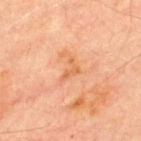Q: Was a biopsy performed?
A: imaged on a skin check; not biopsied
Q: What are the patient's age and sex?
A: male, roughly 70 years of age
Q: How was this image acquired?
A: ~15 mm tile from a whole-body skin photo
Q: Illumination type?
A: cross-polarized
Q: Where on the body is the lesion?
A: the chest